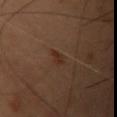notes: catalogued during a skin exam; not biopsied | location: the head or neck | lesion size: about 2.5 mm | illumination: cross-polarized | acquisition: 15 mm crop, total-body photography | subject: female, aged 53–57 | automated lesion analysis: a lesion color around L≈23 a*≈15 b*≈22 in CIELAB and a normalized border contrast of about 6.5; lesion-presence confidence of about 100/100.